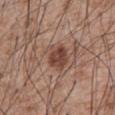Clinical impression: Part of a total-body skin-imaging series; this lesion was reviewed on a skin check and was not flagged for biopsy. Acquisition and patient details: A male subject, about 60 years old. The lesion-visualizer software estimated an automated nevus-likeness rating near 90 out of 100 and a detector confidence of about 100 out of 100 that the crop contains a lesion. This image is a 15 mm lesion crop taken from a total-body photograph. The recorded lesion diameter is about 4 mm. Captured under white-light illumination. The lesion is located on the abdomen.